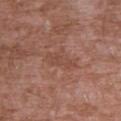The lesion was tiled from a total-body skin photograph and was not biopsied.